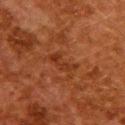{
  "biopsy_status": "not biopsied; imaged during a skin examination",
  "patient": {
    "sex": "female",
    "age_approx": 50
  },
  "automated_metrics": {
    "area_mm2_approx": 4.0,
    "eccentricity": 0.9,
    "vs_skin_contrast_norm": 6.0
  },
  "lighting": "cross-polarized",
  "site": "upper back",
  "image": {
    "source": "total-body photography crop",
    "field_of_view_mm": 15
  }
}